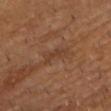• notes: catalogued during a skin exam; not biopsied
• automated metrics: an outline eccentricity of about 0.9 (0 = round, 1 = elongated); a lesion-detection confidence of about 95/100
• image: ~15 mm crop, total-body skin-cancer survey
• lighting: cross-polarized
• subject: male, in their mid- to late 80s or older
• site: the chest
• size: ≈3 mm